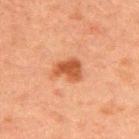follow-up: total-body-photography surveillance lesion; no biopsy | anatomic site: the back | acquisition: 15 mm crop, total-body photography | patient: male, roughly 50 years of age.